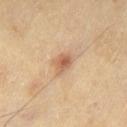Q: Is there a histopathology result?
A: no biopsy performed (imaged during a skin exam)
Q: What lighting was used for the tile?
A: cross-polarized illumination
Q: Lesion size?
A: ≈3 mm
Q: How was this image acquired?
A: total-body-photography crop, ~15 mm field of view
Q: What are the patient's age and sex?
A: male, aged around 65
Q: Automated lesion metrics?
A: a footprint of about 4.5 mm², an outline eccentricity of about 0.7 (0 = round, 1 = elongated), and a shape-asymmetry score of about 0.35 (0 = symmetric); an average lesion color of about L≈61 a*≈21 b*≈34 (CIELAB) and a normalized lesion–skin contrast near 7
Q: Lesion location?
A: the leg